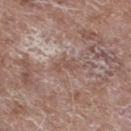This lesion was catalogued during total-body skin photography and was not selected for biopsy. From the leg. Cropped from a total-body skin-imaging series; the visible field is about 15 mm. The subject is a male roughly 60 years of age.